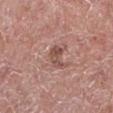Part of a total-body skin-imaging series; this lesion was reviewed on a skin check and was not flagged for biopsy.
The total-body-photography lesion software estimated a footprint of about 4 mm², an eccentricity of roughly 0.8, and a symmetry-axis asymmetry near 0.4.
A 15 mm close-up tile from a total-body photography series done for melanoma screening.
About 3 mm across.
A male subject roughly 75 years of age.
On the left lower leg.
The tile uses white-light illumination.